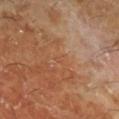Notes:
– workup: catalogued during a skin exam; not biopsied
– lesion diameter: ≈1 mm
– acquisition: ~15 mm tile from a whole-body skin photo
– illumination: cross-polarized illumination
– patient: male, aged approximately 60
– anatomic site: the right lower leg
– TBP lesion metrics: a classifier nevus-likeness of about 0/100 and a detector confidence of about 85 out of 100 that the crop contains a lesion